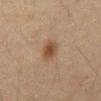Captured during whole-body skin photography for melanoma surveillance; the lesion was not biopsied. Automated tile analysis of the lesion measured a shape eccentricity near 0.6. The software also gave an average lesion color of about L≈39 a*≈15 b*≈27 (CIELAB), a lesion–skin lightness drop of about 9, and a normalized lesion–skin contrast near 8. And it measured border irregularity of about 1.5 on a 0–10 scale, a within-lesion color-variation index near 3/10, and radial color variation of about 1. Captured under cross-polarized illumination. From the abdomen. A male subject in their mid- to late 60s. Cropped from a whole-body photographic skin survey; the tile spans about 15 mm.